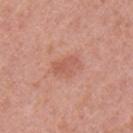No biopsy was performed on this lesion — it was imaged during a full skin examination and was not determined to be concerning. Located on the left upper arm. A lesion tile, about 15 mm wide, cut from a 3D total-body photograph. The patient is a female aged around 40. The recorded lesion diameter is about 3.5 mm.